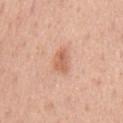notes — imaged on a skin check; not biopsied
lesion size — ~3.5 mm (longest diameter)
illumination — white-light illumination
body site — the chest
acquisition — ~15 mm crop, total-body skin-cancer survey
subject — male, approximately 50 years of age